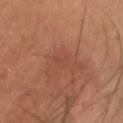Findings:
• follow-up — catalogued during a skin exam; not biopsied
• image — 15 mm crop, total-body photography
• automated lesion analysis — a lesion color around L≈45 a*≈24 b*≈29 in CIELAB, about 4 CIELAB-L* units darker than the surrounding skin, and a normalized border contrast of about 3; a nevus-likeness score of about 0/100 and a detector confidence of about 90 out of 100 that the crop contains a lesion
• location — the head or neck
• illumination — cross-polarized
• patient — male, in their mid-50s